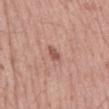biopsy status: no biopsy performed (imaged during a skin exam) | image: total-body-photography crop, ~15 mm field of view | image-analysis metrics: a lesion color around L≈54 a*≈24 b*≈26 in CIELAB, about 10 CIELAB-L* units darker than the surrounding skin, and a lesion-to-skin contrast of about 7 (normalized; higher = more distinct); an automated nevus-likeness rating near 65 out of 100 | patient: male, in their mid- to late 50s | tile lighting: white-light illumination | anatomic site: the mid back | lesion size: ≈2.5 mm.